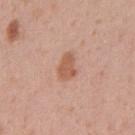  biopsy_status: not biopsied; imaged during a skin examination